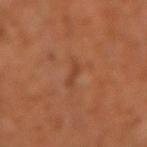Background:
The tile uses cross-polarized illumination. A 15 mm close-up extracted from a 3D total-body photography capture. Automated image analysis of the tile measured a lesion area of about 2 mm², a shape eccentricity near 0.95, and a shape-asymmetry score of about 0.55 (0 = symmetric). The analysis additionally found a border-irregularity index near 7/10, a within-lesion color-variation index near 0/10, and radial color variation of about 0. Located on the left forearm. A male subject, approximately 60 years of age.